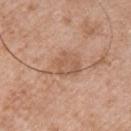biopsy status: total-body-photography surveillance lesion; no biopsy | lesion size: ≈4.5 mm | patient: male, in their 50s | anatomic site: the chest | image: ~15 mm crop, total-body skin-cancer survey | tile lighting: white-light.